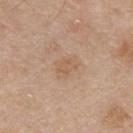Captured during whole-body skin photography for melanoma surveillance; the lesion was not biopsied. The total-body-photography lesion software estimated a footprint of about 5 mm² and an eccentricity of roughly 0.7. The software also gave a border-irregularity index near 3/10 and peripheral color asymmetry of about 1. And it measured an automated nevus-likeness rating near 0 out of 100 and lesion-presence confidence of about 100/100. The subject is a male about 55 years old. Measured at roughly 3 mm in maximum diameter. A lesion tile, about 15 mm wide, cut from a 3D total-body photograph. Imaged with white-light lighting. The lesion is on the chest.